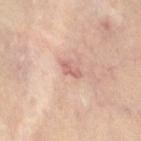| key | value |
|---|---|
| image | ~15 mm crop, total-body skin-cancer survey |
| lighting | cross-polarized illumination |
| lesion size | ≈2.5 mm |
| anatomic site | the right thigh |
| patient | female, about 40 years old |